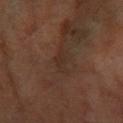Impression:
This lesion was catalogued during total-body skin photography and was not selected for biopsy.
Background:
The patient is a female in their 70s. This image is a 15 mm lesion crop taken from a total-body photograph. On the left forearm.